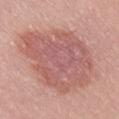Notes:
– follow-up — total-body-photography surveillance lesion; no biopsy
– size — about 10.5 mm
– image source — ~15 mm tile from a whole-body skin photo
– patient — male, aged 38–42
– site — the lower back
– tile lighting — white-light
– TBP lesion metrics — an outline eccentricity of about 0.75 (0 = round, 1 = elongated) and a symmetry-axis asymmetry near 0.25; a mean CIELAB color near L≈58 a*≈25 b*≈24, roughly 10 lightness units darker than nearby skin, and a normalized lesion–skin contrast near 6.5; a border-irregularity index near 4/10, a within-lesion color-variation index near 4/10, and a peripheral color-asymmetry measure near 1